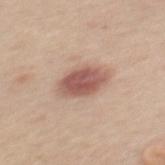follow-up = catalogued during a skin exam; not biopsied | subject = female, aged around 55 | lighting = white-light illumination | anatomic site = the mid back | acquisition = total-body-photography crop, ~15 mm field of view.